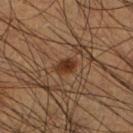Part of a total-body skin-imaging series; this lesion was reviewed on a skin check and was not flagged for biopsy. Located on the right lower leg. A 15 mm close-up extracted from a 3D total-body photography capture. A male patient, in their mid-40s. Longest diameter approximately 2.5 mm. Imaged with cross-polarized lighting.